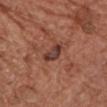TBP lesion metrics = a shape eccentricity near 0.7; a mean CIELAB color near L≈38 a*≈22 b*≈24, roughly 10 lightness units darker than nearby skin, and a normalized lesion–skin contrast near 9.5; border irregularity of about 3.5 on a 0–10 scale, internal color variation of about 3 on a 0–10 scale, and a peripheral color-asymmetry measure near 1
subject = female, roughly 75 years of age
diameter = ~3 mm (longest diameter)
image = ~15 mm crop, total-body skin-cancer survey
anatomic site = the front of the torso